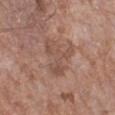Assessment: The lesion was tiled from a total-body skin photograph and was not biopsied. Context: On the left lower leg. Cropped from a total-body skin-imaging series; the visible field is about 15 mm. The total-body-photography lesion software estimated an area of roughly 11 mm², a shape eccentricity near 0.7, and two-axis asymmetry of about 0.65. And it measured a border-irregularity index near 8.5/10, a within-lesion color-variation index near 3/10, and a peripheral color-asymmetry measure near 1. The subject is a female approximately 75 years of age. Longest diameter approximately 5 mm. Captured under white-light illumination.